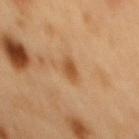| key | value |
|---|---|
| follow-up | imaged on a skin check; not biopsied |
| patient | male, roughly 50 years of age |
| lighting | cross-polarized illumination |
| site | the mid back |
| lesion size | ≈3 mm |
| acquisition | total-body-photography crop, ~15 mm field of view |